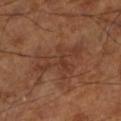The lesion was tiled from a total-body skin photograph and was not biopsied.
Automated tile analysis of the lesion measured an area of roughly 16 mm², a shape eccentricity near 0.8, and a symmetry-axis asymmetry near 0.55. The software also gave a border-irregularity index near 7.5/10 and internal color variation of about 3.5 on a 0–10 scale.
The patient is in their mid-60s.
Longest diameter approximately 7 mm.
Located on the left lower leg.
This image is a 15 mm lesion crop taken from a total-body photograph.
This is a cross-polarized tile.